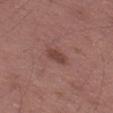Q: Is there a histopathology result?
A: total-body-photography surveillance lesion; no biopsy
Q: Illumination type?
A: white-light illumination
Q: Who is the patient?
A: male, in their 50s
Q: What kind of image is this?
A: ~15 mm tile from a whole-body skin photo
Q: Where on the body is the lesion?
A: the left thigh